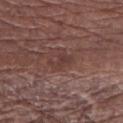Impression: Recorded during total-body skin imaging; not selected for excision or biopsy. Background: About 3 mm across. A 15 mm close-up extracted from a 3D total-body photography capture. The lesion-visualizer software estimated two-axis asymmetry of about 0.35. The analysis additionally found a mean CIELAB color near L≈38 a*≈20 b*≈21, roughly 6 lightness units darker than nearby skin, and a normalized border contrast of about 5.5. The software also gave a border-irregularity rating of about 4/10, a color-variation rating of about 1.5/10, and peripheral color asymmetry of about 0.5. A male subject approximately 65 years of age. Captured under white-light illumination. The lesion is located on the arm.